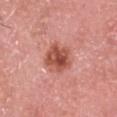Longest diameter approximately 4 mm. A male patient in their 70s. The tile uses white-light illumination. On the head or neck. Cropped from a whole-body photographic skin survey; the tile spans about 15 mm.